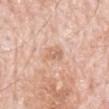| field | value |
|---|---|
| biopsy status | imaged on a skin check; not biopsied |
| lighting | white-light |
| body site | the back |
| image source | 15 mm crop, total-body photography |
| diameter | about 2.5 mm |
| subject | male, approximately 80 years of age |